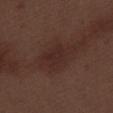Background:
This is a white-light tile. The lesion is on the right thigh. Measured at roughly 5 mm in maximum diameter. A 15 mm crop from a total-body photograph taken for skin-cancer surveillance. The patient is a male roughly 70 years of age. Automated tile analysis of the lesion measured an area of roughly 10 mm², an eccentricity of roughly 0.8, and a shape-asymmetry score of about 0.4 (0 = symmetric). The analysis additionally found a lesion color around L≈26 a*≈18 b*≈20 in CIELAB, about 5 CIELAB-L* units darker than the surrounding skin, and a normalized border contrast of about 6. The software also gave a color-variation rating of about 2/10 and radial color variation of about 0.5.